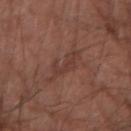Assessment:
Captured during whole-body skin photography for melanoma surveillance; the lesion was not biopsied.
Clinical summary:
Measured at roughly 4.5 mm in maximum diameter. The patient is a male aged approximately 65. The lesion is on the right forearm. A lesion tile, about 15 mm wide, cut from a 3D total-body photograph.